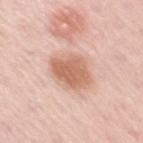Clinical summary: This is a white-light tile. An algorithmic analysis of the crop reported a border-irregularity rating of about 2/10, a within-lesion color-variation index near 3/10, and radial color variation of about 1. The software also gave an automated nevus-likeness rating near 90 out of 100 and a lesion-detection confidence of about 100/100. This image is a 15 mm lesion crop taken from a total-body photograph. A female subject approximately 50 years of age. The lesion is on the arm. The lesion's longest dimension is about 5 mm.